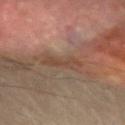Part of a total-body skin-imaging series; this lesion was reviewed on a skin check and was not flagged for biopsy. On the left forearm. A male patient, aged 68–72. Cropped from a total-body skin-imaging series; the visible field is about 15 mm.